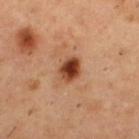follow-up: total-body-photography surveillance lesion; no biopsy
patient: male, aged 53 to 57
tile lighting: cross-polarized
site: the upper back
image: ~15 mm tile from a whole-body skin photo